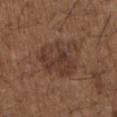The lesion was tiled from a total-body skin photograph and was not biopsied. The patient is a male roughly 50 years of age. A roughly 15 mm field-of-view crop from a total-body skin photograph. From the left upper arm. The lesion's longest dimension is about 5.5 mm.